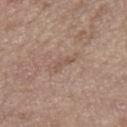| key | value |
|---|---|
| notes | no biopsy performed (imaged during a skin exam) |
| patient | female, in their mid- to late 60s |
| imaging modality | total-body-photography crop, ~15 mm field of view |
| location | the leg |